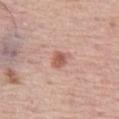| key | value |
|---|---|
| workup | total-body-photography surveillance lesion; no biopsy |
| image | total-body-photography crop, ~15 mm field of view |
| subject | male, aged around 75 |
| tile lighting | white-light illumination |
| location | the left thigh |
| size | ~2.5 mm (longest diameter) |